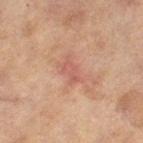Assessment: The lesion was photographed on a routine skin check and not biopsied; there is no pathology result. Background: The tile uses cross-polarized illumination. On the left thigh. A 15 mm crop from a total-body photograph taken for skin-cancer surveillance. The lesion-visualizer software estimated about 6 CIELAB-L* units darker than the surrounding skin and a normalized lesion–skin contrast near 5. And it measured a color-variation rating of about 0/10. It also reported a classifier nevus-likeness of about 0/100 and a detector confidence of about 100 out of 100 that the crop contains a lesion. A female subject aged 58 to 62.